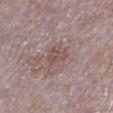{"biopsy_status": "not biopsied; imaged during a skin examination", "site": "left lower leg", "lesion_size": {"long_diameter_mm_approx": 2.5}, "lighting": "white-light", "automated_metrics": {"border_irregularity_0_10": 4.0, "color_variation_0_10": 3.5}, "image": {"source": "total-body photography crop", "field_of_view_mm": 15}, "patient": {"sex": "male", "age_approx": 75}}